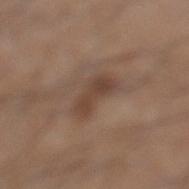Part of a total-body skin-imaging series; this lesion was reviewed on a skin check and was not flagged for biopsy.
The lesion is on the left lower leg.
A region of skin cropped from a whole-body photographic capture, roughly 15 mm wide.
The lesion's longest dimension is about 4 mm.
A male subject roughly 45 years of age.